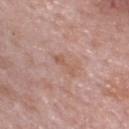follow-up = total-body-photography surveillance lesion; no biopsy | site = the head or neck | image = 15 mm crop, total-body photography | patient = female, aged 83 or older | lighting = white-light | diameter = about 3 mm.